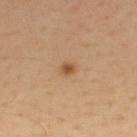follow-up: catalogued during a skin exam; not biopsied | site: the upper back | automated lesion analysis: a lesion color around L≈53 a*≈22 b*≈39 in CIELAB and a normalized border contrast of about 8.5; a classifier nevus-likeness of about 95/100 and lesion-presence confidence of about 100/100 | subject: male, roughly 30 years of age | diameter: ~1.5 mm (longest diameter) | illumination: cross-polarized | image source: ~15 mm tile from a whole-body skin photo.